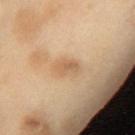Assessment:
Part of a total-body skin-imaging series; this lesion was reviewed on a skin check and was not flagged for biopsy.
Background:
On the chest. A roughly 15 mm field-of-view crop from a total-body skin photograph. A female subject, aged around 55.